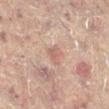Part of a total-body skin-imaging series; this lesion was reviewed on a skin check and was not flagged for biopsy. Imaged with cross-polarized lighting. From the left leg. A female subject about 80 years old. The recorded lesion diameter is about 3 mm. The total-body-photography lesion software estimated a border-irregularity index near 3.5/10, a within-lesion color-variation index near 1.5/10, and radial color variation of about 0.5. The software also gave a classifier nevus-likeness of about 0/100 and a lesion-detection confidence of about 100/100. A roughly 15 mm field-of-view crop from a total-body skin photograph.